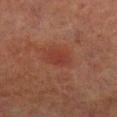workup = no biopsy performed (imaged during a skin exam); subject = male, in their 70s; image source = total-body-photography crop, ~15 mm field of view; site = the right lower leg.